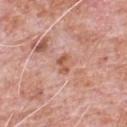Impression:
Recorded during total-body skin imaging; not selected for excision or biopsy.
Background:
The lesion is located on the chest. A roughly 15 mm field-of-view crop from a total-body skin photograph. A male patient aged 78–82. The lesion's longest dimension is about 2.5 mm.